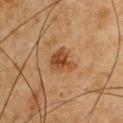Clinical summary:
A male subject, aged approximately 60. A region of skin cropped from a whole-body photographic capture, roughly 15 mm wide. From the chest.